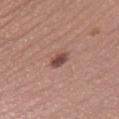Q: Was a biopsy performed?
A: total-body-photography surveillance lesion; no biopsy
Q: Lesion location?
A: the leg
Q: What is the imaging modality?
A: total-body-photography crop, ~15 mm field of view
Q: Automated lesion metrics?
A: a border-irregularity index near 1.5/10, internal color variation of about 3 on a 0–10 scale, and a peripheral color-asymmetry measure near 1
Q: Patient demographics?
A: female, roughly 30 years of age
Q: What is the lesion's diameter?
A: about 2.5 mm